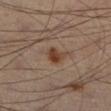The lesion was tiled from a total-body skin photograph and was not biopsied.
An algorithmic analysis of the crop reported an area of roughly 5 mm² and an eccentricity of roughly 0.75. The analysis additionally found a classifier nevus-likeness of about 95/100 and a lesion-detection confidence of about 100/100.
A male patient in their 50s.
Located on the left leg.
Imaged with cross-polarized lighting.
A 15 mm close-up extracted from a 3D total-body photography capture.
Longest diameter approximately 3 mm.